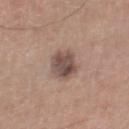This lesion was catalogued during total-body skin photography and was not selected for biopsy. A male subject, aged 63 to 67. On the leg. The total-body-photography lesion software estimated a lesion area of about 8.5 mm² and an outline eccentricity of about 0.65 (0 = round, 1 = elongated). It also reported an average lesion color of about L≈49 a*≈16 b*≈22 (CIELAB) and a normalized border contrast of about 9.5. Captured under white-light illumination. Measured at roughly 3.5 mm in maximum diameter. This image is a 15 mm lesion crop taken from a total-body photograph.